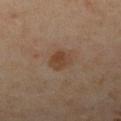Captured during whole-body skin photography for melanoma surveillance; the lesion was not biopsied. About 2.5 mm across. A lesion tile, about 15 mm wide, cut from a 3D total-body photograph. A female subject, aged around 50. Captured under cross-polarized illumination. From the leg.